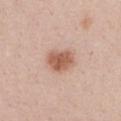Notes:
- patient: female, approximately 40 years of age
- anatomic site: the chest
- image source: ~15 mm crop, total-body skin-cancer survey